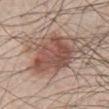Q: Was a biopsy performed?
A: no biopsy performed (imaged during a skin exam)
Q: How was this image acquired?
A: 15 mm crop, total-body photography
Q: How was the tile lit?
A: white-light illumination
Q: Who is the patient?
A: male, aged approximately 50
Q: Lesion location?
A: the chest